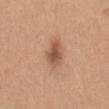Imaged during a routine full-body skin examination; the lesion was not biopsied and no histopathology is available. Approximately 4 mm at its widest. A female subject aged approximately 35. A lesion tile, about 15 mm wide, cut from a 3D total-body photograph. The lesion is on the mid back.